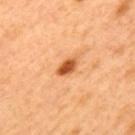The lesion was tiled from a total-body skin photograph and was not biopsied. The patient is a male aged around 50. Approximately 3 mm at its widest. The lesion is located on the back. This is a cross-polarized tile. A roughly 15 mm field-of-view crop from a total-body skin photograph. The lesion-visualizer software estimated an average lesion color of about L≈56 a*≈30 b*≈45 (CIELAB), about 17 CIELAB-L* units darker than the surrounding skin, and a lesion-to-skin contrast of about 10.5 (normalized; higher = more distinct). The software also gave border irregularity of about 2 on a 0–10 scale, a color-variation rating of about 5/10, and radial color variation of about 2. The analysis additionally found a nevus-likeness score of about 95/100 and lesion-presence confidence of about 100/100.